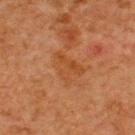No biopsy was performed on this lesion — it was imaged during a full skin examination and was not determined to be concerning. A male subject, in their mid- to late 60s. An algorithmic analysis of the crop reported a mean CIELAB color near L≈41 a*≈23 b*≈36, about 5 CIELAB-L* units darker than the surrounding skin, and a lesion-to-skin contrast of about 5.5 (normalized; higher = more distinct). It also reported a border-irregularity rating of about 5.5/10, a color-variation rating of about 3/10, and a peripheral color-asymmetry measure near 1. This image is a 15 mm lesion crop taken from a total-body photograph. Imaged with cross-polarized lighting. From the upper back.